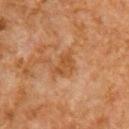{
  "biopsy_status": "not biopsied; imaged during a skin examination",
  "lighting": "cross-polarized",
  "image": {
    "source": "total-body photography crop",
    "field_of_view_mm": 15
  },
  "site": "arm",
  "patient": {
    "sex": "male",
    "age_approx": 80
  }
}